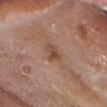A male patient about 65 years old. The lesion-visualizer software estimated a lesion area of about 4.5 mm², an outline eccentricity of about 0.85 (0 = round, 1 = elongated), and two-axis asymmetry of about 0.25. And it measured a border-irregularity rating of about 3/10 and a color-variation rating of about 5.5/10. Located on the head or neck. Cropped from a whole-body photographic skin survey; the tile spans about 15 mm. Captured under white-light illumination.